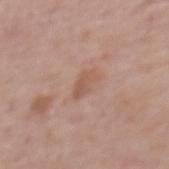lighting=white-light illumination
body site=the back
subject=male, in their 70s
image source=~15 mm crop, total-body skin-cancer survey